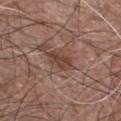  biopsy_status: not biopsied; imaged during a skin examination
  image:
    source: total-body photography crop
    field_of_view_mm: 15
  site: chest
  patient:
    sex: male
    age_approx: 50
  lighting: white-light